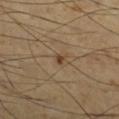Imaged during a routine full-body skin examination; the lesion was not biopsied and no histopathology is available. The total-body-photography lesion software estimated an outline eccentricity of about 0.6 (0 = round, 1 = elongated) and a shape-asymmetry score of about 0.35 (0 = symmetric). The software also gave a lesion color around L≈42 a*≈17 b*≈31 in CIELAB, a lesion–skin lightness drop of about 10, and a lesion-to-skin contrast of about 8 (normalized; higher = more distinct). The analysis additionally found a nevus-likeness score of about 75/100 and a lesion-detection confidence of about 100/100. Located on the right lower leg. The tile uses cross-polarized illumination. This image is a 15 mm lesion crop taken from a total-body photograph. The patient is a male aged approximately 55.